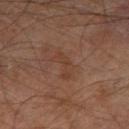- notes: no biopsy performed (imaged during a skin exam)
- tile lighting: cross-polarized
- image source: ~15 mm crop, total-body skin-cancer survey
- location: the right leg
- diameter: about 3.5 mm
- patient: male, about 60 years old